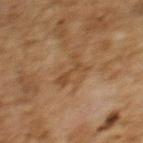Findings:
- workup — no biopsy performed (imaged during a skin exam)
- subject — female, aged 58–62
- illumination — cross-polarized
- lesion diameter — about 3.5 mm
- anatomic site — the upper back
- imaging modality — ~15 mm tile from a whole-body skin photo